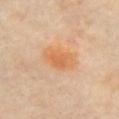  biopsy_status: not biopsied; imaged during a skin examination
  patient:
    sex: female
    age_approx: 60
  image:
    source: total-body photography crop
    field_of_view_mm: 15
  site: chest
  lighting: cross-polarized
  lesion_size:
    long_diameter_mm_approx: 4.0